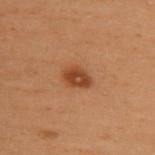{"biopsy_status": "not biopsied; imaged during a skin examination", "patient": {"sex": "female", "age_approx": 50}, "image": {"source": "total-body photography crop", "field_of_view_mm": 15}, "site": "back"}